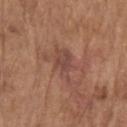<lesion>
  <biopsy_status>not biopsied; imaged during a skin examination</biopsy_status>
  <patient>
    <sex>male</sex>
    <age_approx>80</age_approx>
  </patient>
  <lighting>white-light</lighting>
  <lesion_size>
    <long_diameter_mm_approx>4.0</long_diameter_mm_approx>
  </lesion_size>
  <site>left upper arm</site>
  <automated_metrics>
    <eccentricity>0.8</eccentricity>
    <shape_asymmetry>0.35</shape_asymmetry>
    <vs_skin_darker_L>7.0</vs_skin_darker_L>
    <vs_skin_contrast_norm>6.5</vs_skin_contrast_norm>
    <border_irregularity_0_10>4.0</border_irregularity_0_10>
    <color_variation_0_10>3.0</color_variation_0_10>
    <peripheral_color_asymmetry>1.0</peripheral_color_asymmetry>
  </automated_metrics>
  <image>
    <source>total-body photography crop</source>
    <field_of_view_mm>15</field_of_view_mm>
  </image>
</lesion>